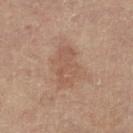Impression: The lesion was tiled from a total-body skin photograph and was not biopsied. Background: A lesion tile, about 15 mm wide, cut from a 3D total-body photograph. The lesion's longest dimension is about 5 mm. Captured under cross-polarized illumination. The subject is a female aged approximately 80. The lesion is located on the left leg.